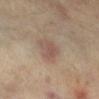Q: Was a biopsy performed?
A: total-body-photography surveillance lesion; no biopsy
Q: Patient demographics?
A: approximately 60 years of age
Q: Where on the body is the lesion?
A: the leg
Q: How was this image acquired?
A: ~15 mm crop, total-body skin-cancer survey
Q: Lesion size?
A: about 3 mm